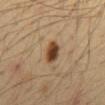Assessment:
Part of a total-body skin-imaging series; this lesion was reviewed on a skin check and was not flagged for biopsy.
Background:
The lesion is located on the abdomen. The tile uses cross-polarized illumination. Measured at roughly 3 mm in maximum diameter. Cropped from a whole-body photographic skin survey; the tile spans about 15 mm. A male patient approximately 35 years of age.